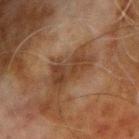Q: Was a biopsy performed?
A: imaged on a skin check; not biopsied
Q: How large is the lesion?
A: ~5.5 mm (longest diameter)
Q: Automated lesion metrics?
A: a lesion color around L≈32 a*≈16 b*≈26 in CIELAB, roughly 8 lightness units darker than nearby skin, and a normalized border contrast of about 7.5; a border-irregularity index near 5/10, a within-lesion color-variation index near 3.5/10, and radial color variation of about 1; a nevus-likeness score of about 0/100 and a detector confidence of about 100 out of 100 that the crop contains a lesion
Q: How was this image acquired?
A: ~15 mm crop, total-body skin-cancer survey
Q: Patient demographics?
A: male, aged approximately 70
Q: What is the anatomic site?
A: the arm